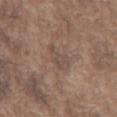Recorded during total-body skin imaging; not selected for excision or biopsy. This is a white-light tile. Automated tile analysis of the lesion measured a footprint of about 4.5 mm², a shape eccentricity near 0.85, and two-axis asymmetry of about 0.6. The software also gave a mean CIELAB color near L≈48 a*≈15 b*≈23, about 6 CIELAB-L* units darker than the surrounding skin, and a normalized border contrast of about 5. The software also gave a within-lesion color-variation index near 2/10 and a peripheral color-asymmetry measure near 0.5. The analysis additionally found a classifier nevus-likeness of about 0/100. Cropped from a whole-body photographic skin survey; the tile spans about 15 mm. The subject is a male in their mid- to late 70s. From the chest. Longest diameter approximately 3.5 mm.